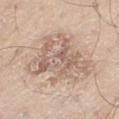notes: imaged on a skin check; not biopsied
size: about 8.5 mm
body site: the left thigh
patient: male, roughly 60 years of age
acquisition: ~15 mm tile from a whole-body skin photo
tile lighting: white-light